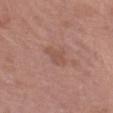Q: Is there a histopathology result?
A: catalogued during a skin exam; not biopsied
Q: What is the lesion's diameter?
A: ~3 mm (longest diameter)
Q: What did automated image analysis measure?
A: a mean CIELAB color near L≈51 a*≈21 b*≈26, a lesion–skin lightness drop of about 6, and a normalized border contrast of about 5
Q: Patient demographics?
A: female, roughly 40 years of age
Q: Lesion location?
A: the abdomen
Q: How was this image acquired?
A: 15 mm crop, total-body photography
Q: Illumination type?
A: white-light